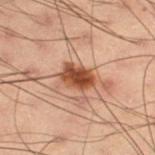No biopsy was performed on this lesion — it was imaged during a full skin examination and was not determined to be concerning. On the left thigh. A male patient, in their mid-50s. A close-up tile cropped from a whole-body skin photograph, about 15 mm across. The total-body-photography lesion software estimated a footprint of about 8.5 mm² and a shape eccentricity near 0.7. The software also gave roughly 13 lightness units darker than nearby skin. The analysis additionally found a nevus-likeness score of about 90/100 and a detector confidence of about 100 out of 100 that the crop contains a lesion. Measured at roughly 4 mm in maximum diameter. The tile uses cross-polarized illumination.